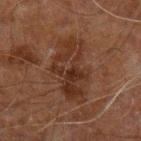notes: total-body-photography surveillance lesion; no biopsy
imaging modality: ~15 mm tile from a whole-body skin photo
site: the left upper arm
subject: male, aged 58–62
size: about 7.5 mm